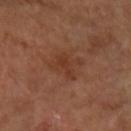biopsy status=imaged on a skin check; not biopsied
body site=the right forearm
lesion size=about 3.5 mm
patient=female, aged 58 to 62
image=~15 mm tile from a whole-body skin photo
lighting=cross-polarized
automated lesion analysis=an average lesion color of about L≈38 a*≈23 b*≈31 (CIELAB) and a normalized border contrast of about 6; a nevus-likeness score of about 0/100 and a detector confidence of about 100 out of 100 that the crop contains a lesion